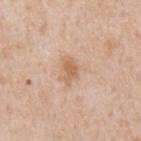Clinical impression: The lesion was photographed on a routine skin check and not biopsied; there is no pathology result. Clinical summary: A close-up tile cropped from a whole-body skin photograph, about 15 mm across. The subject is a male aged 63 to 67. The recorded lesion diameter is about 3 mm. The lesion is located on the mid back.